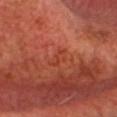Clinical impression: The lesion was photographed on a routine skin check and not biopsied; there is no pathology result. Image and clinical context: An algorithmic analysis of the crop reported a mean CIELAB color near L≈41 a*≈33 b*≈35, about 5 CIELAB-L* units darker than the surrounding skin, and a normalized border contrast of about 5. Captured under cross-polarized illumination. Located on the head or neck. Approximately 3 mm at its widest. The subject is a male about 60 years old. A lesion tile, about 15 mm wide, cut from a 3D total-body photograph.